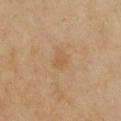{
  "biopsy_status": "not biopsied; imaged during a skin examination",
  "automated_metrics": {
    "border_irregularity_0_10": 2.5
  },
  "lighting": "cross-polarized",
  "site": "chest",
  "lesion_size": {
    "long_diameter_mm_approx": 2.5
  },
  "image": {
    "source": "total-body photography crop",
    "field_of_view_mm": 15
  },
  "patient": {
    "sex": "female",
    "age_approx": 40
  }
}